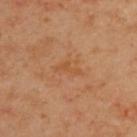  patient:
    sex: male
    age_approx: 45
  image:
    source: total-body photography crop
    field_of_view_mm: 15
  lighting: cross-polarized
  site: back
  lesion_size:
    long_diameter_mm_approx: 3.0